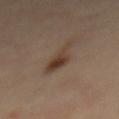| key | value |
|---|---|
| biopsy status | total-body-photography surveillance lesion; no biopsy |
| acquisition | ~15 mm crop, total-body skin-cancer survey |
| subject | female, approximately 60 years of age |
| illumination | cross-polarized illumination |
| body site | the mid back |
| automated metrics | an area of roughly 6.5 mm² and a symmetry-axis asymmetry near 0.45; an automated nevus-likeness rating near 90 out of 100 and a detector confidence of about 100 out of 100 that the crop contains a lesion |